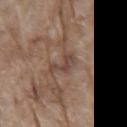{"biopsy_status": "not biopsied; imaged during a skin examination", "site": "mid back", "lighting": "white-light", "patient": {"sex": "male", "age_approx": 70}, "image": {"source": "total-body photography crop", "field_of_view_mm": 15}, "lesion_size": {"long_diameter_mm_approx": 3.5}}